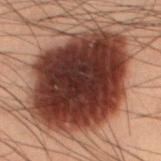Impression:
This lesion was catalogued during total-body skin photography and was not selected for biopsy.
Clinical summary:
Automated image analysis of the tile measured an area of roughly 80 mm², an outline eccentricity of about 0.75 (0 = round, 1 = elongated), and a shape-asymmetry score of about 0.15 (0 = symmetric). The analysis additionally found internal color variation of about 7 on a 0–10 scale and peripheral color asymmetry of about 1.5. It also reported a detector confidence of about 100 out of 100 that the crop contains a lesion. The lesion is located on the leg. Measured at roughly 12 mm in maximum diameter. A region of skin cropped from a whole-body photographic capture, roughly 15 mm wide. Captured under cross-polarized illumination. A male patient, in their mid-50s.